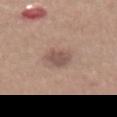workup: imaged on a skin check; not biopsied
lesion diameter: about 3.5 mm
image source: total-body-photography crop, ~15 mm field of view
anatomic site: the abdomen
image-analysis metrics: an area of roughly 6.5 mm² and a shape-asymmetry score of about 0.2 (0 = symmetric); a mean CIELAB color near L≈52 a*≈18 b*≈23, a lesion–skin lightness drop of about 10, and a lesion-to-skin contrast of about 7 (normalized; higher = more distinct); a peripheral color-asymmetry measure near 0.5
patient: female, aged 33 to 37
lighting: white-light illumination